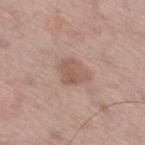The lesion was photographed on a routine skin check and not biopsied; there is no pathology result.
Imaged with white-light lighting.
Measured at roughly 3.5 mm in maximum diameter.
A 15 mm crop from a total-body photograph taken for skin-cancer surveillance.
A male patient aged 68–72.
The total-body-photography lesion software estimated a lesion color around L≈56 a*≈19 b*≈26 in CIELAB, roughly 9 lightness units darker than nearby skin, and a lesion-to-skin contrast of about 6 (normalized; higher = more distinct). And it measured border irregularity of about 2.5 on a 0–10 scale, a within-lesion color-variation index near 2.5/10, and radial color variation of about 1.
From the right thigh.